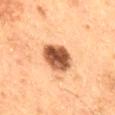Q: Is there a histopathology result?
A: imaged on a skin check; not biopsied
Q: Lesion size?
A: ≈4 mm
Q: What kind of image is this?
A: ~15 mm crop, total-body skin-cancer survey
Q: Patient demographics?
A: male, aged around 60